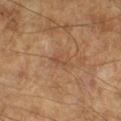{"biopsy_status": "not biopsied; imaged during a skin examination", "image": {"source": "total-body photography crop", "field_of_view_mm": 15}, "lesion_size": {"long_diameter_mm_approx": 2.5}, "patient": {"sex": "male", "age_approx": 65}}